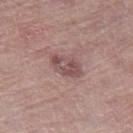Clinical impression: The lesion was photographed on a routine skin check and not biopsied; there is no pathology result. Acquisition and patient details: About 4 mm across. A close-up tile cropped from a whole-body skin photograph, about 15 mm across. A male patient, in their mid- to late 60s. This is a white-light tile. The lesion is on the right thigh.